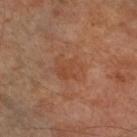anatomic site: the right lower leg; image source: ~15 mm tile from a whole-body skin photo; patient: male, approximately 70 years of age.